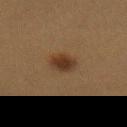Recorded during total-body skin imaging; not selected for excision or biopsy.
Cropped from a whole-body photographic skin survey; the tile spans about 15 mm.
Longest diameter approximately 3 mm.
Captured under cross-polarized illumination.
A female subject, in their 40s.
The lesion is located on the abdomen.
An algorithmic analysis of the crop reported an average lesion color of about L≈28 a*≈14 b*≈25 (CIELAB) and a lesion-to-skin contrast of about 9 (normalized; higher = more distinct). And it measured a border-irregularity rating of about 1.5/10, internal color variation of about 3.5 on a 0–10 scale, and a peripheral color-asymmetry measure near 1.